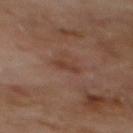notes: imaged on a skin check; not biopsied | image: 15 mm crop, total-body photography | lesion size: about 2.5 mm | lighting: cross-polarized | body site: the mid back | patient: male, aged approximately 70.